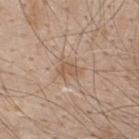subject=male, aged around 50; site=the back; acquisition=~15 mm tile from a whole-body skin photo.